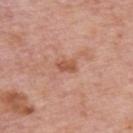Q: Illumination type?
A: white-light
Q: How was this image acquired?
A: ~15 mm tile from a whole-body skin photo
Q: Patient demographics?
A: male, in their mid-70s
Q: How large is the lesion?
A: about 2.5 mm
Q: Lesion location?
A: the upper back